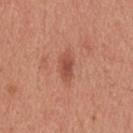workup — catalogued during a skin exam; not biopsied
subject — male, aged 28–32
image — total-body-photography crop, ~15 mm field of view
anatomic site — the head or neck